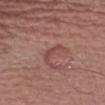The lesion was tiled from a total-body skin photograph and was not biopsied.
A 15 mm close-up tile from a total-body photography series done for melanoma screening.
The lesion is located on the left upper arm.
A male subject aged 63–67.